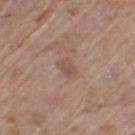tile lighting: white-light | patient: male, about 70 years old | diameter: ~2.5 mm (longest diameter) | imaging modality: ~15 mm tile from a whole-body skin photo | automated metrics: a lesion area of about 3.5 mm²; a mean CIELAB color near L≈52 a*≈18 b*≈26, a lesion–skin lightness drop of about 7, and a normalized lesion–skin contrast near 5.5 | location: the left thigh.